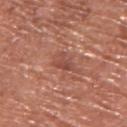The lesion was photographed on a routine skin check and not biopsied; there is no pathology result.